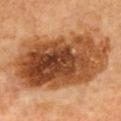Impression:
Imaged during a routine full-body skin examination; the lesion was not biopsied and no histopathology is available.
Background:
A 15 mm close-up tile from a total-body photography series done for melanoma screening. A male subject, aged 58 to 62. The lesion-visualizer software estimated border irregularity of about 3 on a 0–10 scale, internal color variation of about 10 on a 0–10 scale, and radial color variation of about 3.5. It also reported a nevus-likeness score of about 90/100 and lesion-presence confidence of about 100/100. The tile uses cross-polarized illumination. About 14 mm across. From the mid back.